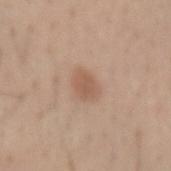The lesion was photographed on a routine skin check and not biopsied; there is no pathology result. A male patient aged approximately 55. Automated tile analysis of the lesion measured a footprint of about 6 mm², an outline eccentricity of about 0.6 (0 = round, 1 = elongated), and a symmetry-axis asymmetry near 0.2. A 15 mm crop from a total-body photograph taken for skin-cancer surveillance. Located on the left forearm. Approximately 3 mm at its widest.